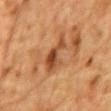Part of a total-body skin-imaging series; this lesion was reviewed on a skin check and was not flagged for biopsy.
The lesion is located on the front of the torso.
Automated image analysis of the tile measured a lesion area of about 7.5 mm² and a symmetry-axis asymmetry near 0.45. The software also gave a lesion color around L≈41 a*≈22 b*≈34 in CIELAB, roughly 11 lightness units darker than nearby skin, and a normalized border contrast of about 8.5. The software also gave an automated nevus-likeness rating near 10 out of 100.
Imaged with cross-polarized lighting.
A male patient, aged around 85 or older.
A region of skin cropped from a whole-body photographic capture, roughly 15 mm wide.
Measured at roughly 5 mm in maximum diameter.